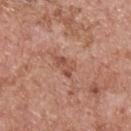The lesion was photographed on a routine skin check and not biopsied; there is no pathology result. This is a white-light tile. From the upper back. Approximately 3 mm at its widest. A 15 mm close-up extracted from a 3D total-body photography capture. The lesion-visualizer software estimated a lesion area of about 4.5 mm², a shape eccentricity near 0.8, and a symmetry-axis asymmetry near 0.45. The analysis additionally found roughly 9 lightness units darker than nearby skin and a normalized lesion–skin contrast near 6. A male subject, about 60 years old.